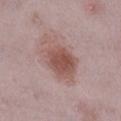Q: Is there a histopathology result?
A: total-body-photography surveillance lesion; no biopsy
Q: Patient demographics?
A: female, roughly 30 years of age
Q: What is the lesion's diameter?
A: ~6 mm (longest diameter)
Q: Lesion location?
A: the leg
Q: How was this image acquired?
A: total-body-photography crop, ~15 mm field of view
Q: How was the tile lit?
A: white-light illumination